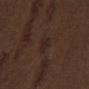Findings:
– workup: no biopsy performed (imaged during a skin exam)
– lesion diameter: about 2.5 mm
– body site: the mid back
– tile lighting: white-light
– patient: male, in their 70s
– imaging modality: total-body-photography crop, ~15 mm field of view